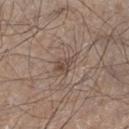Notes:
- workup: total-body-photography surveillance lesion; no biopsy
- lesion diameter: ≈3 mm
- tile lighting: white-light
- acquisition: total-body-photography crop, ~15 mm field of view
- anatomic site: the right lower leg
- patient: male, aged approximately 45
- automated lesion analysis: a lesion–skin lightness drop of about 8; a border-irregularity rating of about 3.5/10, a within-lesion color-variation index near 2.5/10, and peripheral color asymmetry of about 1